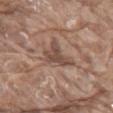Part of a total-body skin-imaging series; this lesion was reviewed on a skin check and was not flagged for biopsy. Measured at roughly 4 mm in maximum diameter. The subject is a male aged 78–82. Imaged with white-light lighting. The lesion is on the mid back. A 15 mm crop from a total-body photograph taken for skin-cancer surveillance. The total-body-photography lesion software estimated a lesion area of about 7 mm², an outline eccentricity of about 0.75 (0 = round, 1 = elongated), and a symmetry-axis asymmetry near 0.6. It also reported a border-irregularity index near 6.5/10. The software also gave an automated nevus-likeness rating near 0 out of 100 and a detector confidence of about 70 out of 100 that the crop contains a lesion.